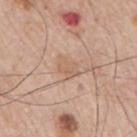biopsy status: no biopsy performed (imaged during a skin exam); image: ~15 mm tile from a whole-body skin photo; patient: male, about 65 years old; body site: the arm; tile lighting: white-light illumination.